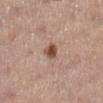Notes:
- image source · 15 mm crop, total-body photography
- site · the leg
- lesion size · ~2.5 mm (longest diameter)
- subject · female, roughly 65 years of age